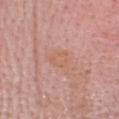patient — female, aged 68–72 | lighting — white-light illumination | anatomic site — the head or neck | image — ~15 mm tile from a whole-body skin photo | automated lesion analysis — an area of roughly 5 mm², an eccentricity of roughly 0.65, and a shape-asymmetry score of about 0.3 (0 = symmetric); a lesion–skin lightness drop of about 4 and a normalized border contrast of about 4.5.